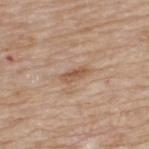| field | value |
|---|---|
| lesion diameter | ≈3 mm |
| illumination | white-light |
| subject | male, aged 63–67 |
| location | the upper back |
| acquisition | ~15 mm crop, total-body skin-cancer survey |